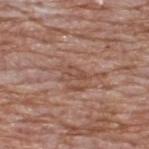follow-up: catalogued during a skin exam; not biopsied | TBP lesion metrics: a lesion area of about 3.5 mm², an outline eccentricity of about 0.85 (0 = round, 1 = elongated), and a shape-asymmetry score of about 0.45 (0 = symmetric); border irregularity of about 4.5 on a 0–10 scale, a within-lesion color-variation index near 1.5/10, and peripheral color asymmetry of about 0.5 | patient: male, aged 58 to 62 | anatomic site: the upper back | image: ~15 mm tile from a whole-body skin photo | tile lighting: white-light | lesion size: ~3 mm (longest diameter).